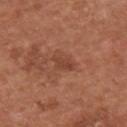Assessment: Imaged during a routine full-body skin examination; the lesion was not biopsied and no histopathology is available. Context: On the upper back. A male subject aged approximately 65. About 3 mm across. Cropped from a total-body skin-imaging series; the visible field is about 15 mm. An algorithmic analysis of the crop reported a mean CIELAB color near L≈43 a*≈25 b*≈30 and a lesion-to-skin contrast of about 6 (normalized; higher = more distinct).